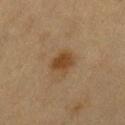This lesion was catalogued during total-body skin photography and was not selected for biopsy. On the left thigh. The total-body-photography lesion software estimated a shape eccentricity near 0.55 and a shape-asymmetry score of about 0.15 (0 = symmetric). It also reported a lesion color around L≈37 a*≈15 b*≈30 in CIELAB, a lesion–skin lightness drop of about 8, and a lesion-to-skin contrast of about 8.5 (normalized; higher = more distinct). It also reported a nevus-likeness score of about 95/100. A female patient, approximately 40 years of age. Approximately 3 mm at its widest. A 15 mm close-up extracted from a 3D total-body photography capture. Imaged with cross-polarized lighting.